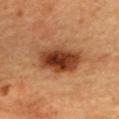  biopsy_status: not biopsied; imaged during a skin examination
  lesion_size:
    long_diameter_mm_approx: 6.5
  patient:
    sex: female
    age_approx: 60
  automated_metrics:
    eccentricity: 0.85
    cielab_L: 44
    cielab_a: 27
    cielab_b: 36
    vs_skin_darker_L: 18.0
    vs_skin_contrast_norm: 12.5
    nevus_likeness_0_100: 100
    lesion_detection_confidence_0_100: 100
  lighting: cross-polarized
  site: upper back
  image:
    source: total-body photography crop
    field_of_view_mm: 15